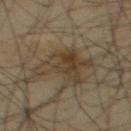{"biopsy_status": "not biopsied; imaged during a skin examination", "lighting": "cross-polarized", "automated_metrics": {"eccentricity": 0.8, "shape_asymmetry": 0.4, "cielab_L": 34, "cielab_a": 9, "cielab_b": 25, "vs_skin_darker_L": 7.0, "border_irregularity_0_10": 6.5, "color_variation_0_10": 4.5, "peripheral_color_asymmetry": 1.5}, "image": {"source": "total-body photography crop", "field_of_view_mm": 15}, "site": "upper back", "patient": {"sex": "male", "age_approx": 35}}